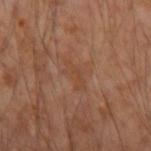Findings:
- workup — no biopsy performed (imaged during a skin exam)
- lighting — cross-polarized illumination
- location — the right forearm
- subject — male, aged approximately 55
- imaging modality — total-body-photography crop, ~15 mm field of view
- automated metrics — an area of roughly 4.5 mm², an outline eccentricity of about 0.85 (0 = round, 1 = elongated), and a symmetry-axis asymmetry near 0.45; a border-irregularity index near 5/10 and internal color variation of about 0.5 on a 0–10 scale; a lesion-detection confidence of about 100/100
- lesion size — ~3.5 mm (longest diameter)